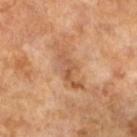Assessment: Imaged during a routine full-body skin examination; the lesion was not biopsied and no histopathology is available. Background: A male subject about 65 years old. The tile uses cross-polarized illumination. This image is a 15 mm lesion crop taken from a total-body photograph. Measured at roughly 6 mm in maximum diameter.